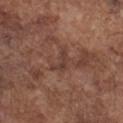Q: Is there a histopathology result?
A: no biopsy performed (imaged during a skin exam)
Q: Where on the body is the lesion?
A: the chest
Q: Who is the patient?
A: male, aged 73 to 77
Q: What lighting was used for the tile?
A: white-light
Q: How was this image acquired?
A: ~15 mm crop, total-body skin-cancer survey